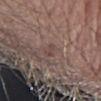This lesion was catalogued during total-body skin photography and was not selected for biopsy. Measured at roughly 2.5 mm in maximum diameter. A male subject in their 80s. This is a white-light tile. A lesion tile, about 15 mm wide, cut from a 3D total-body photograph. From the right forearm.